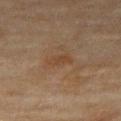Impression: No biopsy was performed on this lesion — it was imaged during a full skin examination and was not determined to be concerning. Background: This is a cross-polarized tile. The recorded lesion diameter is about 3 mm. The lesion is located on the right thigh. A 15 mm close-up extracted from a 3D total-body photography capture. A female subject approximately 60 years of age.